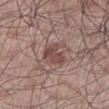Recorded during total-body skin imaging; not selected for excision or biopsy.
Imaged with white-light lighting.
Located on the left lower leg.
The lesion-visualizer software estimated a footprint of about 7 mm², a shape eccentricity near 0.5, and two-axis asymmetry of about 0.3. And it measured a border-irregularity rating of about 3.5/10, internal color variation of about 2.5 on a 0–10 scale, and a peripheral color-asymmetry measure near 1. And it measured a nevus-likeness score of about 5/100 and a lesion-detection confidence of about 100/100.
A 15 mm crop from a total-body photograph taken for skin-cancer surveillance.
Measured at roughly 3.5 mm in maximum diameter.
A male patient, about 55 years old.